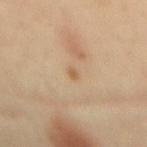imaging modality: ~15 mm crop, total-body skin-cancer survey | image-analysis metrics: an area of roughly 1 mm² and a shape-asymmetry score of about 0.25 (0 = symmetric); a mean CIELAB color near L≈58 a*≈17 b*≈37 and about 8 CIELAB-L* units darker than the surrounding skin; a nevus-likeness score of about 0/100 and a lesion-detection confidence of about 100/100 | illumination: cross-polarized illumination | body site: the back | lesion diameter: about 1.5 mm | subject: female, aged approximately 40.